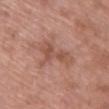Recorded during total-body skin imaging; not selected for excision or biopsy. Imaged with white-light lighting. Located on the upper back. An algorithmic analysis of the crop reported a lesion area of about 9 mm², an eccentricity of roughly 0.85, and a symmetry-axis asymmetry near 0.6. The software also gave a mean CIELAB color near L≈52 a*≈23 b*≈27, a lesion–skin lightness drop of about 8, and a normalized border contrast of about 6. A 15 mm crop from a total-body photograph taken for skin-cancer surveillance. Approximately 5 mm at its widest. The subject is a female roughly 65 years of age.